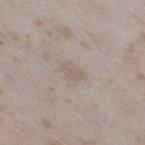Assessment:
Imaged during a routine full-body skin examination; the lesion was not biopsied and no histopathology is available.
Background:
Longest diameter approximately 2.5 mm. The total-body-photography lesion software estimated border irregularity of about 2 on a 0–10 scale, a within-lesion color-variation index near 1/10, and peripheral color asymmetry of about 0.5. A region of skin cropped from a whole-body photographic capture, roughly 15 mm wide. Imaged with white-light lighting. The lesion is located on the right thigh. The subject is a female about 25 years old.